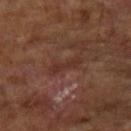Findings:
• follow-up · catalogued during a skin exam; not biopsied
• lesion size · ~4 mm (longest diameter)
• image-analysis metrics · a classifier nevus-likeness of about 0/100 and a lesion-detection confidence of about 70/100
• body site · the arm
• subject · male, aged around 65
• image · ~15 mm tile from a whole-body skin photo